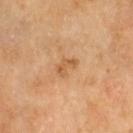Part of a total-body skin-imaging series; this lesion was reviewed on a skin check and was not flagged for biopsy.